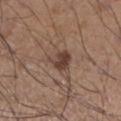workup: total-body-photography surveillance lesion; no biopsy
automated metrics: about 10 CIELAB-L* units darker than the surrounding skin and a lesion-to-skin contrast of about 8.5 (normalized; higher = more distinct); a nevus-likeness score of about 95/100 and a lesion-detection confidence of about 100/100
patient: male, aged 33 to 37
illumination: white-light illumination
body site: the leg
image source: ~15 mm crop, total-body skin-cancer survey